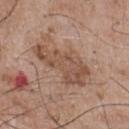* follow-up · imaged on a skin check; not biopsied